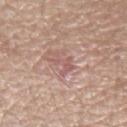Findings:
- notes · total-body-photography surveillance lesion; no biopsy
- illumination · white-light
- subject · male, in their mid-50s
- image · total-body-photography crop, ~15 mm field of view
- anatomic site · the left forearm
- automated metrics · an area of roughly 3.5 mm² and a shape-asymmetry score of about 0.65 (0 = symmetric); an average lesion color of about L≈57 a*≈22 b*≈22 (CIELAB), about 8 CIELAB-L* units darker than the surrounding skin, and a normalized lesion–skin contrast near 5.5; a classifier nevus-likeness of about 0/100 and a detector confidence of about 85 out of 100 that the crop contains a lesion
- lesion size · ~3 mm (longest diameter)